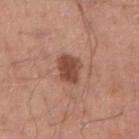  biopsy_status: not biopsied; imaged during a skin examination
  patient:
    sex: male
    age_approx: 30
  automated_metrics:
    area_mm2_approx: 7.0
    eccentricity: 0.75
    shape_asymmetry: 0.25
  site: right upper arm
  lesion_size:
    long_diameter_mm_approx: 3.5
  image:
    source: total-body photography crop
    field_of_view_mm: 15
  lighting: white-light